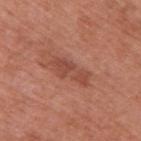{"image": {"source": "total-body photography crop", "field_of_view_mm": 15}, "lighting": "white-light", "patient": {"sex": "male", "age_approx": 70}, "site": "back", "automated_metrics": {"border_irregularity_0_10": 5.0, "color_variation_0_10": 2.5, "peripheral_color_asymmetry": 1.0, "lesion_detection_confidence_0_100": 100}, "lesion_size": {"long_diameter_mm_approx": 5.0}}